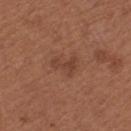Assessment:
Captured during whole-body skin photography for melanoma surveillance; the lesion was not biopsied.
Background:
Captured under white-light illumination. A female subject, aged 53–57. Measured at roughly 3 mm in maximum diameter. From the leg. A lesion tile, about 15 mm wide, cut from a 3D total-body photograph.